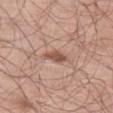• notes · total-body-photography surveillance lesion; no biopsy